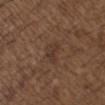Impression: Part of a total-body skin-imaging series; this lesion was reviewed on a skin check and was not flagged for biopsy. Background: The subject is a male about 50 years old. A region of skin cropped from a whole-body photographic capture, roughly 15 mm wide. The lesion is on the chest.